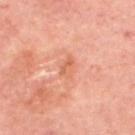Assessment: The lesion was photographed on a routine skin check and not biopsied; there is no pathology result. Background: A roughly 15 mm field-of-view crop from a total-body skin photograph. The patient is aged 53–57. From the upper back.